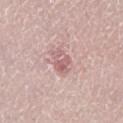Findings:
* follow-up: catalogued during a skin exam; not biopsied
* body site: the leg
* size: about 3 mm
* tile lighting: white-light
* subject: female, roughly 30 years of age
* acquisition: 15 mm crop, total-body photography